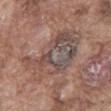{
  "biopsy_status": "not biopsied; imaged during a skin examination",
  "lighting": "white-light",
  "patient": {
    "sex": "male",
    "age_approx": 75
  },
  "image": {
    "source": "total-body photography crop",
    "field_of_view_mm": 15
  },
  "automated_metrics": {
    "area_mm2_approx": 31.0,
    "eccentricity": 0.75,
    "shape_asymmetry": 0.35,
    "color_variation_0_10": 9.0,
    "nevus_likeness_0_100": 0,
    "lesion_detection_confidence_0_100": 50
  },
  "lesion_size": {
    "long_diameter_mm_approx": 8.0
  },
  "site": "abdomen"
}